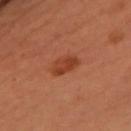  site: chest
  image:
    source: total-body photography crop
    field_of_view_mm: 15
  patient:
    sex: female
    age_approx: 50
  automated_metrics:
    vs_skin_darker_L: 8.0